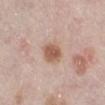Imaged during a routine full-body skin examination; the lesion was not biopsied and no histopathology is available. The patient is a female aged around 65. The lesion is located on the right lower leg. The total-body-photography lesion software estimated a lesion area of about 6.5 mm², a shape eccentricity near 0.6, and a symmetry-axis asymmetry near 0.2. It also reported a lesion color around L≈58 a*≈20 b*≈28 in CIELAB and a lesion-to-skin contrast of about 8.5 (normalized; higher = more distinct). The software also gave a detector confidence of about 100 out of 100 that the crop contains a lesion. Approximately 3.5 mm at its widest. This is a white-light tile. Cropped from a total-body skin-imaging series; the visible field is about 15 mm.